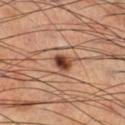follow-up — catalogued during a skin exam; not biopsied
site — the right lower leg
image source — ~15 mm tile from a whole-body skin photo
patient — male, aged 63–67
lighting — cross-polarized illumination
automated lesion analysis — a footprint of about 4 mm², an eccentricity of roughly 0.55, and a symmetry-axis asymmetry near 0.25; an average lesion color of about L≈42 a*≈24 b*≈30 (CIELAB), a lesion–skin lightness drop of about 17, and a lesion-to-skin contrast of about 12.5 (normalized; higher = more distinct); border irregularity of about 2 on a 0–10 scale, a within-lesion color-variation index near 7/10, and a peripheral color-asymmetry measure near 2.5; an automated nevus-likeness rating near 100 out of 100 and lesion-presence confidence of about 100/100